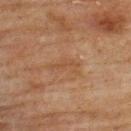{"biopsy_status": "not biopsied; imaged during a skin examination", "lesion_size": {"long_diameter_mm_approx": 3.5}, "site": "upper back", "automated_metrics": {"area_mm2_approx": 4.0, "eccentricity": 0.9, "shape_asymmetry": 0.45, "nevus_likeness_0_100": 0, "lesion_detection_confidence_0_100": 95}, "image": {"source": "total-body photography crop", "field_of_view_mm": 15}, "patient": {"sex": "male", "age_approx": 75}, "lighting": "cross-polarized"}